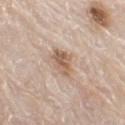Q: What is the imaging modality?
A: ~15 mm crop, total-body skin-cancer survey
Q: Patient demographics?
A: male, aged 78–82
Q: Where on the body is the lesion?
A: the left thigh